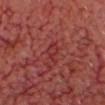follow-up = catalogued during a skin exam; not biopsied | imaging modality = total-body-photography crop, ~15 mm field of view | lesion diameter = ≈3.5 mm | tile lighting = cross-polarized illumination | subject = aged approximately 65 | body site = the head or neck.